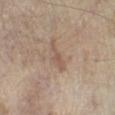{"lesion_size": {"long_diameter_mm_approx": 3.5}, "patient": {"sex": "male", "age_approx": 60}, "image": {"source": "total-body photography crop", "field_of_view_mm": 15}, "lighting": "white-light", "site": "left lower leg"}